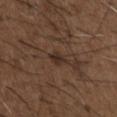workup = total-body-photography surveillance lesion; no biopsy | size = about 3 mm | location = the chest | lighting = white-light | patient = male, approximately 50 years of age | image = 15 mm crop, total-body photography.